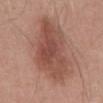image source=~15 mm tile from a whole-body skin photo
lesion size=~9.5 mm (longest diameter)
subject=male, roughly 55 years of age
site=the abdomen